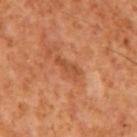Notes:
• patient: male, approximately 65 years of age
• imaging modality: 15 mm crop, total-body photography
• automated metrics: a lesion area of about 4.5 mm² and two-axis asymmetry of about 0.4; border irregularity of about 5 on a 0–10 scale, a color-variation rating of about 1.5/10, and a peripheral color-asymmetry measure near 0.5
• tile lighting: cross-polarized
• size: ~3.5 mm (longest diameter)